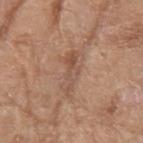Recorded during total-body skin imaging; not selected for excision or biopsy.
A female patient roughly 75 years of age.
The lesion is on the right forearm.
A region of skin cropped from a whole-body photographic capture, roughly 15 mm wide.